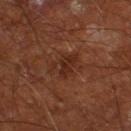{"biopsy_status": "not biopsied; imaged during a skin examination", "image": {"source": "total-body photography crop", "field_of_view_mm": 15}, "automated_metrics": {"area_mm2_approx": 5.5, "eccentricity": 0.8, "cielab_L": 26, "cielab_a": 21, "cielab_b": 26, "vs_skin_darker_L": 6.0, "vs_skin_contrast_norm": 6.5, "border_irregularity_0_10": 3.5, "color_variation_0_10": 2.5, "peripheral_color_asymmetry": 0.5, "lesion_detection_confidence_0_100": 100}, "lesion_size": {"long_diameter_mm_approx": 3.5}, "site": "left thigh", "patient": {"sex": "male", "age_approx": 65}}